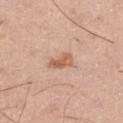No biopsy was performed on this lesion — it was imaged during a full skin examination and was not determined to be concerning. The recorded lesion diameter is about 3 mm. On the right thigh. A male patient, approximately 55 years of age. The tile uses white-light illumination. Automated image analysis of the tile measured a lesion color around L≈60 a*≈22 b*≈32 in CIELAB and a lesion-to-skin contrast of about 7.5 (normalized; higher = more distinct). And it measured border irregularity of about 3 on a 0–10 scale, a within-lesion color-variation index near 3/10, and a peripheral color-asymmetry measure near 1. A lesion tile, about 15 mm wide, cut from a 3D total-body photograph.